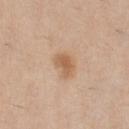  biopsy_status: not biopsied; imaged during a skin examination
  patient:
    sex: male
    age_approx: 35
  automated_metrics:
    vs_skin_darker_L: 9.0
    color_variation_0_10: 2.5
    peripheral_color_asymmetry: 0.5
    nevus_likeness_0_100: 90
    lesion_detection_confidence_0_100: 100
  lesion_size:
    long_diameter_mm_approx: 3.0
  image:
    source: total-body photography crop
    field_of_view_mm: 15
  lighting: white-light
  site: chest